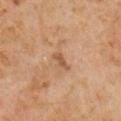The lesion was photographed on a routine skin check and not biopsied; there is no pathology result. A male subject aged 58–62. The recorded lesion diameter is about 2.5 mm. Automated tile analysis of the lesion measured an outline eccentricity of about 0.9 (0 = round, 1 = elongated). It also reported an average lesion color of about L≈50 a*≈20 b*≈33 (CIELAB), roughly 9 lightness units darker than nearby skin, and a normalized border contrast of about 6.5. And it measured a border-irregularity index near 3.5/10, a color-variation rating of about 0/10, and radial color variation of about 0. Imaged with cross-polarized lighting. A lesion tile, about 15 mm wide, cut from a 3D total-body photograph. The lesion is located on the right upper arm.